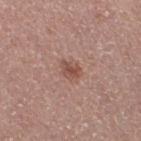biopsy_status: not biopsied; imaged during a skin examination
lighting: white-light
image:
  source: total-body photography crop
  field_of_view_mm: 15
patient:
  sex: female
  age_approx: 50
site: right thigh
automated_metrics:
  nevus_likeness_0_100: 65
lesion_size:
  long_diameter_mm_approx: 2.5